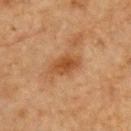No biopsy was performed on this lesion — it was imaged during a full skin examination and was not determined to be concerning. About 3.5 mm across. The patient is a male aged 73–77. This is a cross-polarized tile. The lesion is located on the chest. A 15 mm crop from a total-body photograph taken for skin-cancer surveillance. Automated tile analysis of the lesion measured a mean CIELAB color near L≈39 a*≈20 b*≈33, a lesion–skin lightness drop of about 9, and a normalized lesion–skin contrast near 8. And it measured internal color variation of about 2.5 on a 0–10 scale and peripheral color asymmetry of about 1.